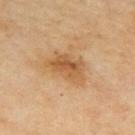Impression: No biopsy was performed on this lesion — it was imaged during a full skin examination and was not determined to be concerning.